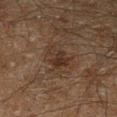Recorded during total-body skin imaging; not selected for excision or biopsy. The lesion is on the left lower leg. An algorithmic analysis of the crop reported an average lesion color of about L≈24 a*≈12 b*≈20 (CIELAB), about 6 CIELAB-L* units darker than the surrounding skin, and a normalized lesion–skin contrast near 6.5. The software also gave a border-irregularity index near 4/10, a within-lesion color-variation index near 4/10, and radial color variation of about 1.5. The analysis additionally found a nevus-likeness score of about 5/100 and a lesion-detection confidence of about 95/100. About 4.5 mm across. The tile uses cross-polarized illumination. A 15 mm crop from a total-body photograph taken for skin-cancer surveillance. The patient is a male aged 58 to 62.